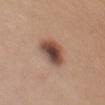Impression: The lesion was tiled from a total-body skin photograph and was not biopsied. Clinical summary: Captured under white-light illumination. About 4.5 mm across. A region of skin cropped from a whole-body photographic capture, roughly 15 mm wide. The lesion is on the chest. The subject is a female aged 43 to 47.